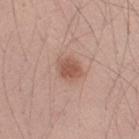Q: Was this lesion biopsied?
A: imaged on a skin check; not biopsied
Q: How large is the lesion?
A: ~3 mm (longest diameter)
Q: Lesion location?
A: the right upper arm
Q: What are the patient's age and sex?
A: male, approximately 25 years of age
Q: How was this image acquired?
A: ~15 mm tile from a whole-body skin photo
Q: How was the tile lit?
A: white-light